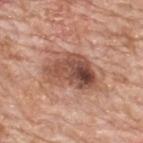This image is a 15 mm lesion crop taken from a total-body photograph. Imaged with white-light lighting. On the upper back. The lesion-visualizer software estimated a footprint of about 18 mm², an eccentricity of roughly 0.8, and a shape-asymmetry score of about 0.3 (0 = symmetric). And it measured about 14 CIELAB-L* units darker than the surrounding skin. It also reported a border-irregularity index near 3/10 and a within-lesion color-variation index near 9.5/10. A male patient, approximately 80 years of age. Approximately 6 mm at its widest.